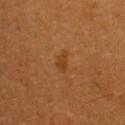Clinical impression:
Imaged during a routine full-body skin examination; the lesion was not biopsied and no histopathology is available.
Image and clinical context:
A female patient, about 55 years old. Automated image analysis of the tile measured an area of roughly 3 mm², an outline eccentricity of about 0.85 (0 = round, 1 = elongated), and two-axis asymmetry of about 0.45. A 15 mm crop from a total-body photograph taken for skin-cancer surveillance. On the upper back. The recorded lesion diameter is about 2.5 mm.